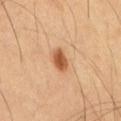From the abdomen.
The subject is a male about 60 years old.
Longest diameter approximately 2.5 mm.
Captured under cross-polarized illumination.
A 15 mm close-up extracted from a 3D total-body photography capture.